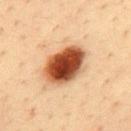Q: Was this lesion biopsied?
A: total-body-photography surveillance lesion; no biopsy
Q: What are the patient's age and sex?
A: male, approximately 35 years of age
Q: What is the imaging modality?
A: 15 mm crop, total-body photography
Q: What did automated image analysis measure?
A: a mean CIELAB color near L≈48 a*≈26 b*≈36, roughly 23 lightness units darker than nearby skin, and a lesion-to-skin contrast of about 15 (normalized; higher = more distinct); internal color variation of about 9 on a 0–10 scale and a peripheral color-asymmetry measure near 2.5; a classifier nevus-likeness of about 100/100 and a detector confidence of about 100 out of 100 that the crop contains a lesion
Q: Where on the body is the lesion?
A: the mid back